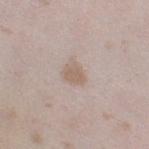Notes:
– follow-up · catalogued during a skin exam; not biopsied
– image · 15 mm crop, total-body photography
– diameter · ≈2.5 mm
– subject · female, approximately 25 years of age
– body site · the right thigh
– tile lighting · white-light